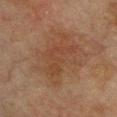This lesion was catalogued during total-body skin photography and was not selected for biopsy. The total-body-photography lesion software estimated a footprint of about 9 mm², an outline eccentricity of about 0.85 (0 = round, 1 = elongated), and a shape-asymmetry score of about 0.65 (0 = symmetric). The software also gave an average lesion color of about L≈35 a*≈18 b*≈26 (CIELAB), a lesion–skin lightness drop of about 5, and a normalized lesion–skin contrast near 4.5. The software also gave an automated nevus-likeness rating near 5 out of 100 and lesion-presence confidence of about 100/100. A male patient, approximately 75 years of age. Measured at roughly 5.5 mm in maximum diameter. From the chest. This image is a 15 mm lesion crop taken from a total-body photograph. This is a cross-polarized tile.